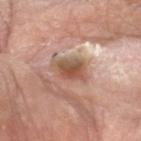Clinical impression:
The lesion was tiled from a total-body skin photograph and was not biopsied.
Image and clinical context:
On the left forearm. A female patient about 75 years old. A 15 mm close-up extracted from a 3D total-body photography capture.